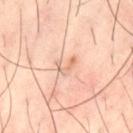follow-up = imaged on a skin check; not biopsied
subject = male, aged 38 to 42
location = the left thigh
acquisition = ~15 mm tile from a whole-body skin photo